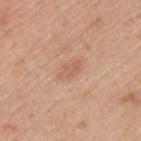notes = catalogued during a skin exam; not biopsied
lesion diameter = about 3 mm
patient = male, aged approximately 45
anatomic site = the left upper arm
lighting = white-light illumination
imaging modality = 15 mm crop, total-body photography